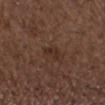  biopsy_status: not biopsied; imaged during a skin examination
  image:
    source: total-body photography crop
    field_of_view_mm: 15
  lesion_size:
    long_diameter_mm_approx: 2.5
  site: arm
  lighting: white-light
  patient:
    sex: male
    age_approx: 50